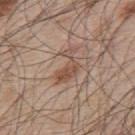follow-up = no biopsy performed (imaged during a skin exam); acquisition = total-body-photography crop, ~15 mm field of view; body site = the upper back; lesion diameter = about 4.5 mm; patient = male, approximately 55 years of age; automated metrics = a border-irregularity index near 7/10, internal color variation of about 2.5 on a 0–10 scale, and peripheral color asymmetry of about 1.